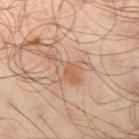workup = total-body-photography surveillance lesion; no biopsy | imaging modality = ~15 mm crop, total-body skin-cancer survey | diameter = ≈5.5 mm | illumination = cross-polarized illumination | TBP lesion metrics = a lesion area of about 9.5 mm², a shape eccentricity near 0.8, and two-axis asymmetry of about 0.5; a lesion–skin lightness drop of about 8 and a normalized lesion–skin contrast near 5.5; border irregularity of about 7 on a 0–10 scale, a within-lesion color-variation index near 5/10, and peripheral color asymmetry of about 1.5; a classifier nevus-likeness of about 0/100 and a detector confidence of about 100 out of 100 that the crop contains a lesion | location = the right thigh | patient = male, in their mid-60s.